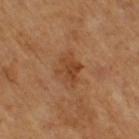Part of a total-body skin-imaging series; this lesion was reviewed on a skin check and was not flagged for biopsy. Captured under cross-polarized illumination. Located on the right thigh. The total-body-photography lesion software estimated a lesion color around L≈45 a*≈24 b*≈36 in CIELAB, about 8 CIELAB-L* units darker than the surrounding skin, and a lesion-to-skin contrast of about 6.5 (normalized; higher = more distinct). And it measured a border-irregularity rating of about 4/10, a color-variation rating of about 3/10, and radial color variation of about 1. A region of skin cropped from a whole-body photographic capture, roughly 15 mm wide. A female patient aged 53 to 57. Approximately 4 mm at its widest.